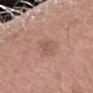Notes:
• biopsy status: total-body-photography surveillance lesion; no biopsy
• automated metrics: a border-irregularity rating of about 3.5/10, a within-lesion color-variation index near 0.5/10, and peripheral color asymmetry of about 0; a classifier nevus-likeness of about 0/100 and a lesion-detection confidence of about 100/100
• image source: total-body-photography crop, ~15 mm field of view
• size: ~3 mm (longest diameter)
• lighting: white-light illumination
• subject: female, aged 63–67
• site: the left forearm